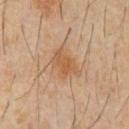No biopsy was performed on this lesion — it was imaged during a full skin examination and was not determined to be concerning.
The lesion is located on the abdomen.
The patient is a male approximately 60 years of age.
A 15 mm close-up extracted from a 3D total-body photography capture.
Captured under cross-polarized illumination.
About 4 mm across.